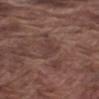Captured during whole-body skin photography for melanoma surveillance; the lesion was not biopsied. The total-body-photography lesion software estimated about 5 CIELAB-L* units darker than the surrounding skin. And it measured a classifier nevus-likeness of about 0/100. Captured under white-light illumination. A roughly 15 mm field-of-view crop from a total-body skin photograph. A male patient about 75 years old. On the right upper arm.